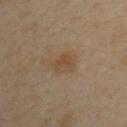patient: male, roughly 50 years of age
size: ≈3.5 mm
tile lighting: cross-polarized
imaging modality: 15 mm crop, total-body photography
location: the arm